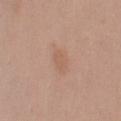Assessment:
The lesion was tiled from a total-body skin photograph and was not biopsied.
Context:
A close-up tile cropped from a whole-body skin photograph, about 15 mm across. Located on the left upper arm. A male patient, aged around 50.